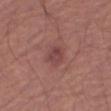Findings:
• workup: total-body-photography surveillance lesion; no biopsy
• body site: the right thigh
• lesion size: about 3 mm
• imaging modality: ~15 mm crop, total-body skin-cancer survey
• subject: male, aged approximately 65
• lighting: white-light illumination